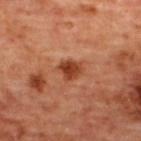No biopsy was performed on this lesion — it was imaged during a full skin examination and was not determined to be concerning. Longest diameter approximately 3 mm. A female patient in their mid-40s. The lesion is located on the upper back. A 15 mm close-up extracted from a 3D total-body photography capture. Captured under cross-polarized illumination.